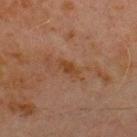| field | value |
|---|---|
| tile lighting | cross-polarized illumination |
| acquisition | ~15 mm tile from a whole-body skin photo |
| body site | the front of the torso |
| patient | male, aged around 70 |
| diameter | ≈3 mm |
| image-analysis metrics | an area of roughly 3.5 mm², an outline eccentricity of about 0.85 (0 = round, 1 = elongated), and a symmetry-axis asymmetry near 0.2; a within-lesion color-variation index near 2/10 and a peripheral color-asymmetry measure near 0.5 |